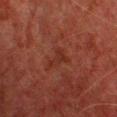A lesion tile, about 15 mm wide, cut from a 3D total-body photograph.
A male patient, aged around 60.
Approximately 3 mm at its widest.
The tile uses cross-polarized illumination.
Located on the chest.